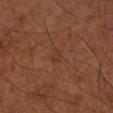This lesion was catalogued during total-body skin photography and was not selected for biopsy. On the right forearm. Measured at roughly 1.5 mm in maximum diameter. A close-up tile cropped from a whole-body skin photograph, about 15 mm across. A male subject aged 53 to 57.